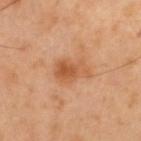* patient — male, approximately 40 years of age
* automated lesion analysis — a shape eccentricity near 0.8 and a shape-asymmetry score of about 0.25 (0 = symmetric)
* location — the back
* tile lighting — cross-polarized
* diameter — ≈4 mm
* acquisition — ~15 mm crop, total-body skin-cancer survey